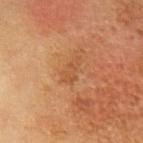Q: Was this lesion biopsied?
A: imaged on a skin check; not biopsied
Q: What is the lesion's diameter?
A: about 3 mm
Q: Patient demographics?
A: female, about 50 years old
Q: Illumination type?
A: cross-polarized illumination
Q: What kind of image is this?
A: ~15 mm crop, total-body skin-cancer survey
Q: What is the anatomic site?
A: the chest